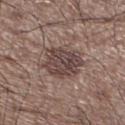<record>
  <biopsy_status>not biopsied; imaged during a skin examination</biopsy_status>
  <image>
    <source>total-body photography crop</source>
    <field_of_view_mm>15</field_of_view_mm>
  </image>
  <lighting>white-light</lighting>
  <site>left lower leg</site>
  <patient>
    <sex>male</sex>
    <age_approx>60</age_approx>
  </patient>
  <lesion_size>
    <long_diameter_mm_approx>5.0</long_diameter_mm_approx>
  </lesion_size>
</record>